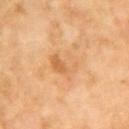  biopsy_status: not biopsied; imaged during a skin examination
  lesion_size:
    long_diameter_mm_approx: 4.0
  image:
    source: total-body photography crop
    field_of_view_mm: 15
  site: left upper arm
  patient:
    sex: female
    age_approx: 70
  automated_metrics:
    area_mm2_approx: 9.5
    eccentricity: 0.7
    shape_asymmetry: 0.3
    cielab_L: 68
    cielab_a: 23
    cielab_b: 43
    vs_skin_darker_L: 7.0
    vs_skin_contrast_norm: 5.0
    nevus_likeness_0_100: 0
    lesion_detection_confidence_0_100: 100